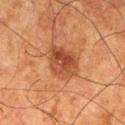Recorded during total-body skin imaging; not selected for excision or biopsy.
The subject is a male approximately 80 years of age.
A close-up tile cropped from a whole-body skin photograph, about 15 mm across.
The lesion is on the leg.